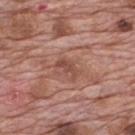Clinical impression:
This lesion was catalogued during total-body skin photography and was not selected for biopsy.
Context:
Approximately 2.5 mm at its widest. The lesion is located on the mid back. This is a white-light tile. The total-body-photography lesion software estimated a border-irregularity index near 5/10, internal color variation of about 0 on a 0–10 scale, and radial color variation of about 0. And it measured a classifier nevus-likeness of about 0/100 and a lesion-detection confidence of about 100/100. A male patient aged around 70. A roughly 15 mm field-of-view crop from a total-body skin photograph.